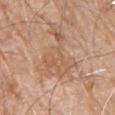follow-up — catalogued during a skin exam; not biopsied
image — total-body-photography crop, ~15 mm field of view
site — the left upper arm
TBP lesion metrics — a footprint of about 20 mm² and an outline eccentricity of about 0.85 (0 = round, 1 = elongated); a color-variation rating of about 3/10 and radial color variation of about 1; a classifier nevus-likeness of about 0/100
subject — male, about 80 years old